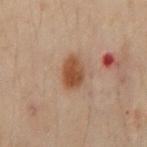biopsy status: catalogued during a skin exam; not biopsied | size: ~4 mm (longest diameter) | location: the chest | patient: male, in their 50s | acquisition: ~15 mm tile from a whole-body skin photo | automated metrics: an area of roughly 7.5 mm², an outline eccentricity of about 0.8 (0 = round, 1 = elongated), and two-axis asymmetry of about 0.15; a lesion color around L≈37 a*≈16 b*≈26 in CIELAB, a lesion–skin lightness drop of about 10, and a normalized border contrast of about 9.5.